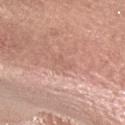biopsy status — imaged on a skin check; not biopsied
patient — female, aged 53–57
body site — the head or neck
imaging modality — total-body-photography crop, ~15 mm field of view
diameter — about 2 mm
lighting — white-light
image-analysis metrics — an area of roughly 2 mm², an eccentricity of roughly 0.85, and a symmetry-axis asymmetry near 0.45; roughly 5 lightness units darker than nearby skin and a normalized border contrast of about 4; a lesion-detection confidence of about 90/100